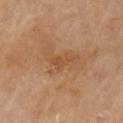biopsy status = catalogued during a skin exam; not biopsied | imaging modality = ~15 mm crop, total-body skin-cancer survey | anatomic site = the left upper arm | patient = male, aged approximately 70.